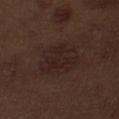Part of a total-body skin-imaging series; this lesion was reviewed on a skin check and was not flagged for biopsy. Approximately 5.5 mm at its widest. The tile uses white-light illumination. A male subject, aged around 70. A region of skin cropped from a whole-body photographic capture, roughly 15 mm wide. From the abdomen.